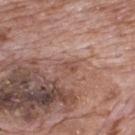No biopsy was performed on this lesion — it was imaged during a full skin examination and was not determined to be concerning.
A male patient, in their 70s.
The lesion is located on the mid back.
Captured under white-light illumination.
The recorded lesion diameter is about 2.5 mm.
Automated image analysis of the tile measured a lesion area of about 3 mm² and a shape eccentricity near 0.8. The analysis additionally found a lesion color around L≈50 a*≈21 b*≈26 in CIELAB, a lesion–skin lightness drop of about 8, and a normalized lesion–skin contrast near 5.5.
A lesion tile, about 15 mm wide, cut from a 3D total-body photograph.